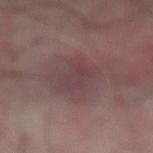Part of a total-body skin-imaging series; this lesion was reviewed on a skin check and was not flagged for biopsy. The lesion is located on the abdomen. A region of skin cropped from a whole-body photographic capture, roughly 15 mm wide. Longest diameter approximately 5 mm. Imaged with cross-polarized lighting. A male patient, roughly 50 years of age. Automated image analysis of the tile measured a footprint of about 9.5 mm², a shape eccentricity near 0.85, and a symmetry-axis asymmetry near 0.4. It also reported a lesion color around L≈39 a*≈18 b*≈15 in CIELAB, about 5 CIELAB-L* units darker than the surrounding skin, and a normalized lesion–skin contrast near 5. The software also gave an automated nevus-likeness rating near 0 out of 100 and a detector confidence of about 95 out of 100 that the crop contains a lesion.